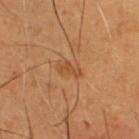Impression:
The lesion was photographed on a routine skin check and not biopsied; there is no pathology result.
Context:
This image is a 15 mm lesion crop taken from a total-body photograph. Imaged with cross-polarized lighting. The lesion is on the chest. The patient is a male approximately 50 years of age. The lesion's longest dimension is about 3 mm. The total-body-photography lesion software estimated a lesion area of about 2.5 mm², an eccentricity of roughly 0.9, and a symmetry-axis asymmetry near 0.45. The software also gave a border-irregularity index near 5/10, a color-variation rating of about 0/10, and radial color variation of about 0.